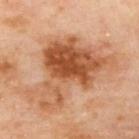Assessment:
The lesion was photographed on a routine skin check and not biopsied; there is no pathology result.
Acquisition and patient details:
A lesion tile, about 15 mm wide, cut from a 3D total-body photograph. Approximately 9.5 mm at its widest. The lesion is located on the upper back. This is a cross-polarized tile. A female patient aged approximately 60.